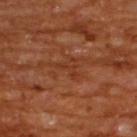Q: Was this lesion biopsied?
A: catalogued during a skin exam; not biopsied
Q: What is the anatomic site?
A: the upper back
Q: How large is the lesion?
A: about 2.5 mm
Q: What is the imaging modality?
A: ~15 mm crop, total-body skin-cancer survey
Q: What are the patient's age and sex?
A: male, approximately 65 years of age